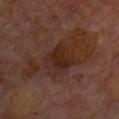follow-up: total-body-photography surveillance lesion; no biopsy | lesion size: ≈4 mm | anatomic site: the front of the torso | image: total-body-photography crop, ~15 mm field of view | subject: female, in their 60s | automated lesion analysis: a lesion color around L≈27 a*≈20 b*≈25 in CIELAB and a lesion-to-skin contrast of about 7 (normalized; higher = more distinct) | tile lighting: cross-polarized.